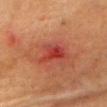notes — catalogued during a skin exam; not biopsied
site — the head or neck
subject — male, aged around 85 or older
acquisition — ~15 mm tile from a whole-body skin photo